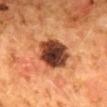workup = no biopsy performed (imaged during a skin exam)
patient = female, roughly 50 years of age
automated metrics = a border-irregularity rating of about 1.5/10, internal color variation of about 7.5 on a 0–10 scale, and radial color variation of about 1.5; an automated nevus-likeness rating near 30 out of 100 and lesion-presence confidence of about 100/100
site = the mid back
imaging modality = ~15 mm crop, total-body skin-cancer survey
tile lighting = cross-polarized illumination
lesion diameter = ≈5 mm